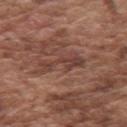Assessment:
This lesion was catalogued during total-body skin photography and was not selected for biopsy.
Clinical summary:
A male patient aged 73 to 77. This image is a 15 mm lesion crop taken from a total-body photograph. An algorithmic analysis of the crop reported border irregularity of about 8 on a 0–10 scale, a color-variation rating of about 3/10, and radial color variation of about 1. On the left upper arm. The tile uses white-light illumination. Longest diameter approximately 5 mm.